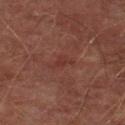Findings:
- workup — total-body-photography surveillance lesion; no biopsy
- tile lighting — cross-polarized illumination
- TBP lesion metrics — a mean CIELAB color near L≈27 a*≈21 b*≈20, roughly 4 lightness units darker than nearby skin, and a lesion-to-skin contrast of about 4.5 (normalized; higher = more distinct); a border-irregularity index near 5/10 and peripheral color asymmetry of about 0
- size — ~2.5 mm (longest diameter)
- imaging modality — ~15 mm tile from a whole-body skin photo
- body site — the left lower leg
- patient — male, approximately 75 years of age